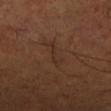Clinical impression: The lesion was photographed on a routine skin check and not biopsied; there is no pathology result. Background: A lesion tile, about 15 mm wide, cut from a 3D total-body photograph. Measured at roughly 3.5 mm in maximum diameter. A male patient, roughly 50 years of age. Located on the leg.